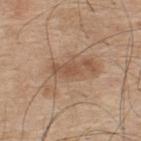Imaged during a routine full-body skin examination; the lesion was not biopsied and no histopathology is available. The tile uses white-light illumination. On the upper back. About 7 mm across. The subject is a male about 75 years old. A 15 mm close-up extracted from a 3D total-body photography capture.